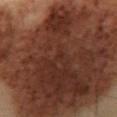The lesion was tiled from a total-body skin photograph and was not biopsied. The total-body-photography lesion software estimated an area of roughly 220 mm², an outline eccentricity of about 0.7 (0 = round, 1 = elongated), and a symmetry-axis asymmetry near 0.3. The analysis additionally found an average lesion color of about L≈27 a*≈17 b*≈22 (CIELAB) and about 13 CIELAB-L* units darker than the surrounding skin. It also reported a border-irregularity index near 4/10, a color-variation rating of about 6/10, and peripheral color asymmetry of about 2. A 15 mm crop from a total-body photograph taken for skin-cancer surveillance. From the abdomen. The subject is a male about 55 years old. About 21 mm across.